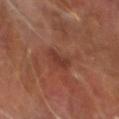  biopsy_status: not biopsied; imaged during a skin examination
  image:
    source: total-body photography crop
    field_of_view_mm: 15
  site: right forearm
  patient:
    sex: male
    age_approx: 65
  automated_metrics:
    eccentricity: 0.75
    cielab_L: 34
    cielab_a: 23
    cielab_b: 26
    vs_skin_darker_L: 7.0
    vs_skin_contrast_norm: 6.5
    border_irregularity_0_10: 4.0
    color_variation_0_10: 3.0